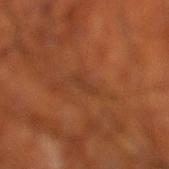Clinical impression: The lesion was tiled from a total-body skin photograph and was not biopsied. Clinical summary: The subject is a male aged 68 to 72. Measured at roughly 3 mm in maximum diameter. This is a cross-polarized tile. The lesion is located on the left lower leg. A roughly 15 mm field-of-view crop from a total-body skin photograph.